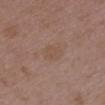<record>
<biopsy_status>not biopsied; imaged during a skin examination</biopsy_status>
<image>
  <source>total-body photography crop</source>
  <field_of_view_mm>15</field_of_view_mm>
</image>
<automated_metrics>
  <cielab_L>50</cielab_L>
  <cielab_a>16</cielab_a>
  <cielab_b>27</cielab_b>
  <vs_skin_darker_L>4.0</vs_skin_darker_L>
  <border_irregularity_0_10>2.5</border_irregularity_0_10>
  <color_variation_0_10>0.5</color_variation_0_10>
  <peripheral_color_asymmetry>0.5</peripheral_color_asymmetry>
  <nevus_likeness_0_100>0</nevus_likeness_0_100>
  <lesion_detection_confidence_0_100>100</lesion_detection_confidence_0_100>
</automated_metrics>
<site>chest</site>
<patient>
  <sex>female</sex>
  <age_approx>35</age_approx>
</patient>
<lighting>white-light</lighting>
<lesion_size>
  <long_diameter_mm_approx>3.5</long_diameter_mm_approx>
</lesion_size>
</record>